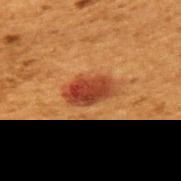Q: Is there a histopathology result?
A: catalogued during a skin exam; not biopsied
Q: Patient demographics?
A: female, aged 48–52
Q: Lesion location?
A: the upper back
Q: What is the lesion's diameter?
A: about 4.5 mm
Q: How was the tile lit?
A: cross-polarized
Q: What is the imaging modality?
A: 15 mm crop, total-body photography